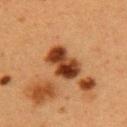The lesion was tiled from a total-body skin photograph and was not biopsied. Automated tile analysis of the lesion measured a footprint of about 12 mm² and an eccentricity of roughly 0.8. Imaged with cross-polarized lighting. The recorded lesion diameter is about 5 mm. A female patient, aged 38–42. A lesion tile, about 15 mm wide, cut from a 3D total-body photograph. Located on the left upper arm.